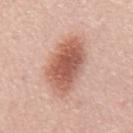follow-up: catalogued during a skin exam; not biopsied
illumination: white-light illumination
site: the mid back
lesion diameter: about 7.5 mm
patient: male, aged 63 to 67
automated lesion analysis: a border-irregularity index near 2.5/10 and internal color variation of about 5 on a 0–10 scale
image source: ~15 mm tile from a whole-body skin photo